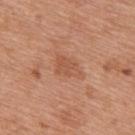follow-up=total-body-photography surveillance lesion; no biopsy | site=the back | imaging modality=~15 mm crop, total-body skin-cancer survey | subject=male, aged 68 to 72.